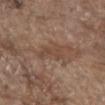The lesion was tiled from a total-body skin photograph and was not biopsied.
Cropped from a total-body skin-imaging series; the visible field is about 15 mm.
The patient is a male aged 78–82.
The lesion's longest dimension is about 4 mm.
The lesion is on the abdomen.
The tile uses white-light illumination.